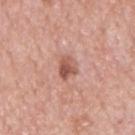Clinical impression: This lesion was catalogued during total-body skin photography and was not selected for biopsy. Image and clinical context: The lesion is on the back. The patient is a male approximately 65 years of age. The lesion-visualizer software estimated a lesion color around L≈54 a*≈26 b*≈27 in CIELAB and a lesion-to-skin contrast of about 8.5 (normalized; higher = more distinct). Measured at roughly 3 mm in maximum diameter. Imaged with white-light lighting. A region of skin cropped from a whole-body photographic capture, roughly 15 mm wide.